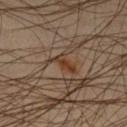Clinical impression: The lesion was photographed on a routine skin check and not biopsied; there is no pathology result. Image and clinical context: A 15 mm close-up tile from a total-body photography series done for melanoma screening. Located on the left lower leg. A male patient approximately 45 years of age.